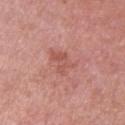biopsy_status: not biopsied; imaged during a skin examination
image:
  source: total-body photography crop
  field_of_view_mm: 15
lighting: white-light
lesion_size:
  long_diameter_mm_approx: 3.0
patient:
  sex: male
  age_approx: 50
site: left upper arm